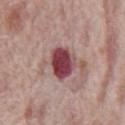The lesion was photographed on a routine skin check and not biopsied; there is no pathology result.
The lesion is on the abdomen.
The recorded lesion diameter is about 4 mm.
Automated image analysis of the tile measured an average lesion color of about L≈43 a*≈29 b*≈17 (CIELAB), about 18 CIELAB-L* units darker than the surrounding skin, and a lesion-to-skin contrast of about 13 (normalized; higher = more distinct). It also reported a border-irregularity index near 2/10, a within-lesion color-variation index near 5/10, and radial color variation of about 1.5.
The patient is a male aged approximately 70.
This image is a 15 mm lesion crop taken from a total-body photograph.